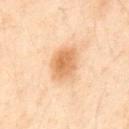Assessment:
Imaged during a routine full-body skin examination; the lesion was not biopsied and no histopathology is available.
Clinical summary:
The subject is a male in their 50s. A 15 mm close-up extracted from a 3D total-body photography capture. An algorithmic analysis of the crop reported an average lesion color of about L≈53 a*≈17 b*≈32 (CIELAB), roughly 9 lightness units darker than nearby skin, and a lesion-to-skin contrast of about 7.5 (normalized; higher = more distinct). The software also gave lesion-presence confidence of about 100/100. The lesion is on the abdomen. The tile uses cross-polarized illumination.